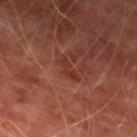Part of a total-body skin-imaging series; this lesion was reviewed on a skin check and was not flagged for biopsy. A male subject in their mid-70s. Cropped from a whole-body photographic skin survey; the tile spans about 15 mm. This is a cross-polarized tile. The recorded lesion diameter is about 3 mm. The lesion is located on the left lower leg.